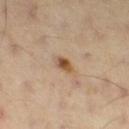Assessment:
No biopsy was performed on this lesion — it was imaged during a full skin examination and was not determined to be concerning.
Acquisition and patient details:
Approximately 2 mm at its widest. The tile uses cross-polarized illumination. A 15 mm close-up extracted from a 3D total-body photography capture. Automated tile analysis of the lesion measured an eccentricity of roughly 0.7 and a symmetry-axis asymmetry near 0.25. The analysis additionally found a mean CIELAB color near L≈52 a*≈19 b*≈34 and a lesion-to-skin contrast of about 9.5 (normalized; higher = more distinct). And it measured a border-irregularity rating of about 2/10 and internal color variation of about 5.5 on a 0–10 scale. The software also gave a classifier nevus-likeness of about 95/100 and a detector confidence of about 100 out of 100 that the crop contains a lesion. A male patient, about 55 years old. Located on the leg.